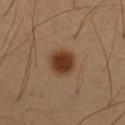Clinical impression: No biopsy was performed on this lesion — it was imaged during a full skin examination and was not determined to be concerning. Context: A male patient aged around 55. A 15 mm close-up extracted from a 3D total-body photography capture. Captured under cross-polarized illumination. The total-body-photography lesion software estimated a lesion area of about 8.5 mm², an outline eccentricity of about 0.5 (0 = round, 1 = elongated), and two-axis asymmetry of about 0.1. And it measured a lesion color around L≈36 a*≈19 b*≈31 in CIELAB. The analysis additionally found a nevus-likeness score of about 100/100 and a lesion-detection confidence of about 100/100. The lesion is on the chest.